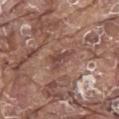Approximately 3 mm at its widest. The patient is a male about 80 years old. A lesion tile, about 15 mm wide, cut from a 3D total-body photograph. On the mid back. The tile uses white-light illumination.